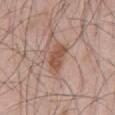From the mid back. A male subject roughly 45 years of age. A lesion tile, about 15 mm wide, cut from a 3D total-body photograph.